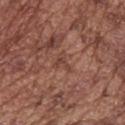Notes:
• follow-up: no biopsy performed (imaged during a skin exam)
• site: the back
• size: ~2.5 mm (longest diameter)
• illumination: white-light
• acquisition: total-body-photography crop, ~15 mm field of view
• subject: male, roughly 75 years of age
• TBP lesion metrics: a lesion color around L≈43 a*≈22 b*≈26 in CIELAB, roughly 7 lightness units darker than nearby skin, and a lesion-to-skin contrast of about 5.5 (normalized; higher = more distinct)